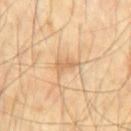Findings:
* lesion size · ≈3 mm
* tile lighting · cross-polarized
* subject · male, aged approximately 70
* acquisition · 15 mm crop, total-body photography
* anatomic site · the chest